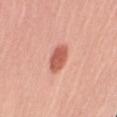{
  "biopsy_status": "not biopsied; imaged during a skin examination"
}